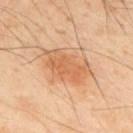Background:
Automated tile analysis of the lesion measured a mean CIELAB color near L≈63 a*≈24 b*≈39, roughly 10 lightness units darker than nearby skin, and a lesion-to-skin contrast of about 6.5 (normalized; higher = more distinct). It also reported a classifier nevus-likeness of about 85/100 and lesion-presence confidence of about 100/100. Cropped from a total-body skin-imaging series; the visible field is about 15 mm. The tile uses cross-polarized illumination. From the upper back. A male patient aged 48 to 52. The recorded lesion diameter is about 6 mm.